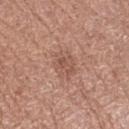| field | value |
|---|---|
| acquisition | ~15 mm tile from a whole-body skin photo |
| size | about 3 mm |
| site | the left lower leg |
| patient | female, about 50 years old |